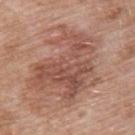Clinical impression: The lesion was photographed on a routine skin check and not biopsied; there is no pathology result. Clinical summary: A roughly 15 mm field-of-view crop from a total-body skin photograph. The recorded lesion diameter is about 9 mm. The patient is a male in their mid-50s. Automated image analysis of the tile measured a border-irregularity rating of about 6.5/10 and internal color variation of about 6 on a 0–10 scale. On the upper back.